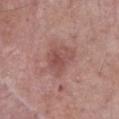Acquisition and patient details:
From the chest. A close-up tile cropped from a whole-body skin photograph, about 15 mm across. A male patient aged approximately 65.